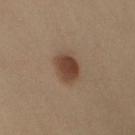• follow-up · imaged on a skin check; not biopsied
• image-analysis metrics · a lesion color around L≈42 a*≈18 b*≈28 in CIELAB and a normalized lesion–skin contrast near 10; a border-irregularity rating of about 2/10, internal color variation of about 4 on a 0–10 scale, and radial color variation of about 1.5
• patient · female, aged around 40
• imaging modality · ~15 mm crop, total-body skin-cancer survey
• location · the left arm